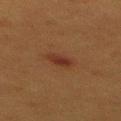Assessment: Part of a total-body skin-imaging series; this lesion was reviewed on a skin check and was not flagged for biopsy. Background: The lesion is located on the upper back. A female subject aged around 40. Cropped from a total-body skin-imaging series; the visible field is about 15 mm.